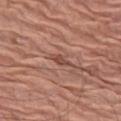Recorded during total-body skin imaging; not selected for excision or biopsy. This is a white-light tile. The lesion-visualizer software estimated an eccentricity of roughly 0.9 and two-axis asymmetry of about 0.2. And it measured a lesion color around L≈48 a*≈24 b*≈27 in CIELAB and roughly 9 lightness units darker than nearby skin. It also reported border irregularity of about 2 on a 0–10 scale and a color-variation rating of about 1.5/10. It also reported an automated nevus-likeness rating near 5 out of 100 and lesion-presence confidence of about 80/100. The lesion's longest dimension is about 2.5 mm. Located on the leg. A close-up tile cropped from a whole-body skin photograph, about 15 mm across. The patient is a female aged approximately 80.